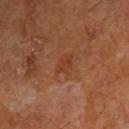Impression: Captured during whole-body skin photography for melanoma surveillance; the lesion was not biopsied. Background: An algorithmic analysis of the crop reported peripheral color asymmetry of about 1. The subject is a male aged around 60. A 15 mm crop from a total-body photograph taken for skin-cancer surveillance. Located on the right upper arm. About 2.5 mm across. This is a cross-polarized tile.